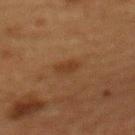An algorithmic analysis of the crop reported a lesion area of about 3.5 mm², an outline eccentricity of about 0.85 (0 = round, 1 = elongated), and a symmetry-axis asymmetry near 0.3. It also reported border irregularity of about 3 on a 0–10 scale, internal color variation of about 1 on a 0–10 scale, and peripheral color asymmetry of about 0.5. It also reported a classifier nevus-likeness of about 65/100.
On the mid back.
The lesion's longest dimension is about 3 mm.
A 15 mm close-up tile from a total-body photography series done for melanoma screening.
Captured under cross-polarized illumination.
A female subject, aged 48 to 52.